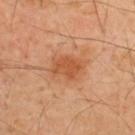The lesion was photographed on a routine skin check and not biopsied; there is no pathology result.
Longest diameter approximately 3.5 mm.
The lesion is on the upper back.
Imaged with cross-polarized lighting.
A male patient, aged 48–52.
A 15 mm crop from a total-body photograph taken for skin-cancer surveillance.